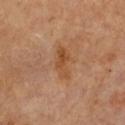Impression:
Captured during whole-body skin photography for melanoma surveillance; the lesion was not biopsied.
Image and clinical context:
From the chest. A female subject aged 63–67. A close-up tile cropped from a whole-body skin photograph, about 15 mm across. Captured under cross-polarized illumination. Approximately 4 mm at its widest. The total-body-photography lesion software estimated an average lesion color of about L≈47 a*≈22 b*≈35 (CIELAB) and a lesion-to-skin contrast of about 6.5 (normalized; higher = more distinct). The software also gave a classifier nevus-likeness of about 0/100.